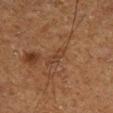Assessment: The lesion was photographed on a routine skin check and not biopsied; there is no pathology result. Context: Captured under cross-polarized illumination. The lesion-visualizer software estimated a border-irregularity rating of about 3/10, a color-variation rating of about 0.5/10, and radial color variation of about 0. A 15 mm close-up tile from a total-body photography series done for melanoma screening. Located on the right lower leg. The subject is a male aged 73–77.